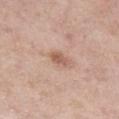The lesion was photographed on a routine skin check and not biopsied; there is no pathology result.
A close-up tile cropped from a whole-body skin photograph, about 15 mm across.
A female patient, aged 53 to 57.
The lesion's longest dimension is about 2.5 mm.
Automated image analysis of the tile measured a mean CIELAB color near L≈58 a*≈20 b*≈29, a lesion–skin lightness drop of about 11, and a lesion-to-skin contrast of about 7 (normalized; higher = more distinct). It also reported lesion-presence confidence of about 100/100.
The lesion is on the right lower leg.
The tile uses white-light illumination.